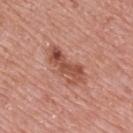Imaged during a routine full-body skin examination; the lesion was not biopsied and no histopathology is available.
A close-up tile cropped from a whole-body skin photograph, about 15 mm across.
A male subject, roughly 70 years of age.
The tile uses white-light illumination.
Located on the back.
The recorded lesion diameter is about 5.5 mm.